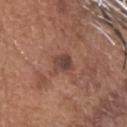Recorded during total-body skin imaging; not selected for excision or biopsy.
A 15 mm close-up tile from a total-body photography series done for melanoma screening.
The recorded lesion diameter is about 3 mm.
An algorithmic analysis of the crop reported a lesion area of about 4.5 mm² and a shape-asymmetry score of about 0.2 (0 = symmetric). The analysis additionally found a lesion color around L≈42 a*≈20 b*≈25 in CIELAB, about 9 CIELAB-L* units darker than the surrounding skin, and a normalized lesion–skin contrast near 8. The analysis additionally found a within-lesion color-variation index near 3.5/10 and radial color variation of about 1.5.
On the head or neck.
Imaged with white-light lighting.
A male subject aged around 75.